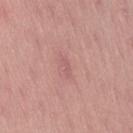Acquisition and patient details: A lesion tile, about 15 mm wide, cut from a 3D total-body photograph. Longest diameter approximately 2.5 mm. The patient is a male aged around 55. The lesion is located on the right thigh. The tile uses white-light illumination.